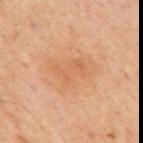biopsy status=no biopsy performed (imaged during a skin exam); body site=the chest; patient=male, about 70 years old; lesion diameter=~5.5 mm (longest diameter); tile lighting=cross-polarized; imaging modality=~15 mm tile from a whole-body skin photo.